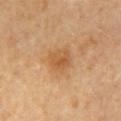Findings:
* follow-up — no biopsy performed (imaged during a skin exam)
* body site — the back
* subject — female, approximately 50 years of age
* acquisition — ~15 mm crop, total-body skin-cancer survey
* lesion size — ~3 mm (longest diameter)